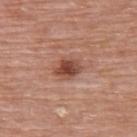follow-up = catalogued during a skin exam; not biopsied | image = ~15 mm tile from a whole-body skin photo | patient = female, aged approximately 65 | site = the chest | size = ≈3 mm.